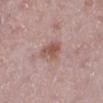Captured during whole-body skin photography for melanoma surveillance; the lesion was not biopsied. The subject is a female approximately 45 years of age. A close-up tile cropped from a whole-body skin photograph, about 15 mm across. Automated image analysis of the tile measured an area of roughly 8 mm² and an eccentricity of roughly 0.7. The analysis additionally found a mean CIELAB color near L≈55 a*≈20 b*≈24 and about 9 CIELAB-L* units darker than the surrounding skin. And it measured border irregularity of about 2.5 on a 0–10 scale, a within-lesion color-variation index near 4.5/10, and a peripheral color-asymmetry measure near 1.5. The software also gave a classifier nevus-likeness of about 50/100. The tile uses white-light illumination. Longest diameter approximately 3.5 mm. Located on the left lower leg.